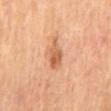Clinical impression: Imaged during a routine full-body skin examination; the lesion was not biopsied and no histopathology is available. Background: Longest diameter approximately 4 mm. This is a cross-polarized tile. A 15 mm close-up tile from a total-body photography series done for melanoma screening. On the mid back. Automated image analysis of the tile measured a border-irregularity index near 3.5/10, a color-variation rating of about 4.5/10, and peripheral color asymmetry of about 1.5. A male patient in their 70s.